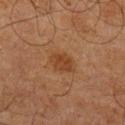– workup: total-body-photography surveillance lesion; no biopsy
– patient: male, aged 63 to 67
– lighting: cross-polarized
– imaging modality: 15 mm crop, total-body photography
– anatomic site: the right lower leg
– size: ~2.5 mm (longest diameter)